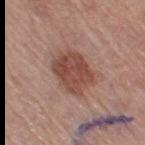Part of a total-body skin-imaging series; this lesion was reviewed on a skin check and was not flagged for biopsy. The recorded lesion diameter is about 5 mm. A female subject, aged around 55. Automated tile analysis of the lesion measured a footprint of about 15 mm², a shape eccentricity near 0.65, and two-axis asymmetry of about 0.15. The software also gave a classifier nevus-likeness of about 55/100 and lesion-presence confidence of about 100/100. Imaged with white-light lighting. The lesion is located on the right thigh. A 15 mm close-up tile from a total-body photography series done for melanoma screening.